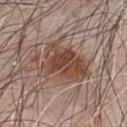The lesion's longest dimension is about 6 mm. This is a white-light tile. The lesion is on the chest. The patient is a male aged 78 to 82. A close-up tile cropped from a whole-body skin photograph, about 15 mm across.